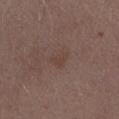Recorded during total-body skin imaging; not selected for excision or biopsy. The patient is a female in their 30s. A 15 mm crop from a total-body photograph taken for skin-cancer surveillance. The lesion is located on the right forearm. Captured under white-light illumination.